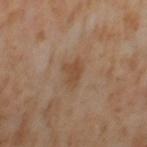biopsy status: total-body-photography surveillance lesion; no biopsy | illumination: cross-polarized | patient: female, aged approximately 55 | image-analysis metrics: a lesion area of about 5 mm², a shape eccentricity near 0.75, and two-axis asymmetry of about 0.4; border irregularity of about 4 on a 0–10 scale, internal color variation of about 1.5 on a 0–10 scale, and a peripheral color-asymmetry measure near 0.5; a classifier nevus-likeness of about 0/100 and a detector confidence of about 100 out of 100 that the crop contains a lesion | location: the left thigh | lesion size: about 3 mm | acquisition: total-body-photography crop, ~15 mm field of view.